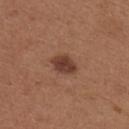Recorded during total-body skin imaging; not selected for excision or biopsy.
This is a white-light tile.
On the upper back.
A female subject aged 28–32.
An algorithmic analysis of the crop reported an eccentricity of roughly 0.7 and two-axis asymmetry of about 0.15. And it measured border irregularity of about 1.5 on a 0–10 scale, internal color variation of about 2.5 on a 0–10 scale, and peripheral color asymmetry of about 1. The analysis additionally found an automated nevus-likeness rating near 75 out of 100.
The lesion's longest dimension is about 3.5 mm.
A 15 mm close-up tile from a total-body photography series done for melanoma screening.